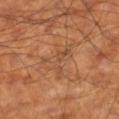{"biopsy_status": "not biopsied; imaged during a skin examination", "image": {"source": "total-body photography crop", "field_of_view_mm": 15}, "automated_metrics": {"border_irregularity_0_10": 10.0, "color_variation_0_10": 2.5, "nevus_likeness_0_100": 0, "lesion_detection_confidence_0_100": 80}, "site": "right lower leg", "lighting": "cross-polarized", "patient": {"sex": "male", "age_approx": 60}, "lesion_size": {"long_diameter_mm_approx": 4.5}}